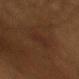Located on the right upper arm. The subject is a male about 60 years old. A 15 mm crop from a total-body photograph taken for skin-cancer surveillance.